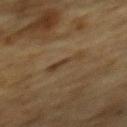Part of a total-body skin-imaging series; this lesion was reviewed on a skin check and was not flagged for biopsy. Cropped from a whole-body photographic skin survey; the tile spans about 15 mm. A male patient aged 83 or older. Longest diameter approximately 3.5 mm. On the mid back. This is a cross-polarized tile.